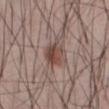No biopsy was performed on this lesion — it was imaged during a full skin examination and was not determined to be concerning.
This is a white-light tile.
Cropped from a whole-body photographic skin survey; the tile spans about 15 mm.
The lesion is located on the front of the torso.
The patient is a male in their mid- to late 50s.